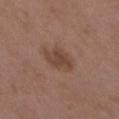A female subject aged 28–32.
The tile uses white-light illumination.
Cropped from a total-body skin-imaging series; the visible field is about 15 mm.
From the left upper arm.